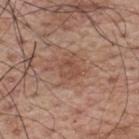Assessment: No biopsy was performed on this lesion — it was imaged during a full skin examination and was not determined to be concerning.